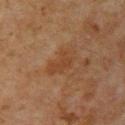notes: imaged on a skin check; not biopsied
acquisition: 15 mm crop, total-body photography
diameter: ≈4 mm
patient: male, about 60 years old
location: the left upper arm